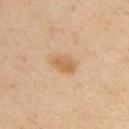workup = imaged on a skin check; not biopsied | patient = male, about 50 years old | imaging modality = ~15 mm tile from a whole-body skin photo | automated metrics = a border-irregularity rating of about 2/10, internal color variation of about 2.5 on a 0–10 scale, and radial color variation of about 1; a nevus-likeness score of about 40/100 and a lesion-detection confidence of about 100/100 | site = the right upper arm.